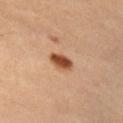Captured during whole-body skin photography for melanoma surveillance; the lesion was not biopsied.
The lesion is located on the right upper arm.
Measured at roughly 3 mm in maximum diameter.
Cropped from a total-body skin-imaging series; the visible field is about 15 mm.
Imaged with cross-polarized lighting.
A female subject aged approximately 45.